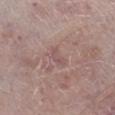Recorded during total-body skin imaging; not selected for excision or biopsy.
Located on the leg.
The subject is a male in their mid-60s.
The tile uses white-light illumination.
Cropped from a total-body skin-imaging series; the visible field is about 15 mm.